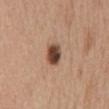biopsy status: total-body-photography surveillance lesion; no biopsy | site: the mid back | image: ~15 mm tile from a whole-body skin photo | subject: female, about 75 years old.